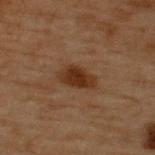The lesion was tiled from a total-body skin photograph and was not biopsied.
A close-up tile cropped from a whole-body skin photograph, about 15 mm across.
A male patient roughly 70 years of age.
The lesion is on the upper back.